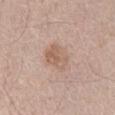Imaged during a routine full-body skin examination; the lesion was not biopsied and no histopathology is available. The lesion-visualizer software estimated a footprint of about 10 mm², an eccentricity of roughly 0.75, and two-axis asymmetry of about 0.2. It also reported a lesion color around L≈60 a*≈17 b*≈27 in CIELAB and roughly 8 lightness units darker than nearby skin. A close-up tile cropped from a whole-body skin photograph, about 15 mm across. About 4.5 mm across. The patient is a male roughly 65 years of age. Located on the abdomen. This is a white-light tile.